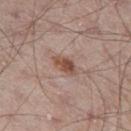follow-up: catalogued during a skin exam; not biopsied
tile lighting: white-light
lesion size: ≈3.5 mm
body site: the left thigh
patient: male, about 65 years old
acquisition: 15 mm crop, total-body photography